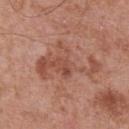Impression:
The lesion was tiled from a total-body skin photograph and was not biopsied.
Acquisition and patient details:
The patient is a male in their 70s. This image is a 15 mm lesion crop taken from a total-body photograph. This is a white-light tile. On the front of the torso. The lesion's longest dimension is about 7.5 mm.